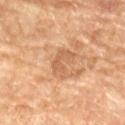The lesion was photographed on a routine skin check and not biopsied; there is no pathology result.
A female patient, approximately 70 years of age.
The lesion's longest dimension is about 3.5 mm.
This is a cross-polarized tile.
Located on the left lower leg.
A 15 mm close-up extracted from a 3D total-body photography capture.